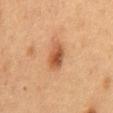No biopsy was performed on this lesion — it was imaged during a full skin examination and was not determined to be concerning. A 15 mm close-up extracted from a 3D total-body photography capture. About 3.5 mm across. A male patient in their mid- to late 50s. The lesion is located on the mid back. This is a cross-polarized tile.